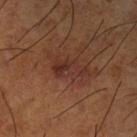follow-up: no biopsy performed (imaged during a skin exam); illumination: cross-polarized illumination; subject: male, in their mid- to late 60s; location: the left lower leg; acquisition: ~15 mm crop, total-body skin-cancer survey; lesion diameter: about 4 mm.